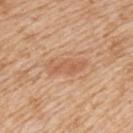Assessment:
Recorded during total-body skin imaging; not selected for excision or biopsy.
Context:
A male subject aged 63 to 67. From the left upper arm. Cropped from a total-body skin-imaging series; the visible field is about 15 mm.